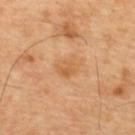<record>
<biopsy_status>not biopsied; imaged during a skin examination</biopsy_status>
<site>upper back</site>
<image>
  <source>total-body photography crop</source>
  <field_of_view_mm>15</field_of_view_mm>
</image>
<lighting>cross-polarized</lighting>
<lesion_size>
  <long_diameter_mm_approx>3.0</long_diameter_mm_approx>
</lesion_size>
<patient>
  <sex>male</sex>
  <age_approx>60</age_approx>
</patient>
</record>